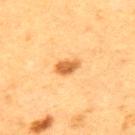Imaged during a routine full-body skin examination; the lesion was not biopsied and no histopathology is available. This is a cross-polarized tile. Longest diameter approximately 2.5 mm. A region of skin cropped from a whole-body photographic capture, roughly 15 mm wide. A male patient aged around 50. From the upper back.